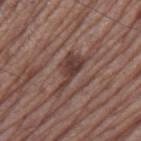Clinical impression:
Part of a total-body skin-imaging series; this lesion was reviewed on a skin check and was not flagged for biopsy.
Clinical summary:
The lesion is on the leg. A male patient in their mid-60s. A 15 mm crop from a total-body photograph taken for skin-cancer surveillance.